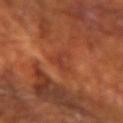Clinical impression:
This lesion was catalogued during total-body skin photography and was not selected for biopsy.
Context:
Automated image analysis of the tile measured a lesion color around L≈38 a*≈28 b*≈32 in CIELAB, a lesion–skin lightness drop of about 6, and a lesion-to-skin contrast of about 5.5 (normalized; higher = more distinct). The software also gave a border-irregularity index near 8/10 and a color-variation rating of about 0/10. It also reported a nevus-likeness score of about 0/100. About 3 mm across. Cropped from a total-body skin-imaging series; the visible field is about 15 mm. The patient is a male aged 68–72. Located on the left forearm. This is a cross-polarized tile.